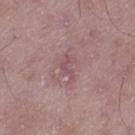Part of a total-body skin-imaging series; this lesion was reviewed on a skin check and was not flagged for biopsy. Longest diameter approximately 2.5 mm. The patient is a male aged 48–52. The lesion-visualizer software estimated a lesion area of about 2.5 mm² and two-axis asymmetry of about 0.6. The analysis additionally found a border-irregularity rating of about 7/10, a color-variation rating of about 0/10, and radial color variation of about 0. And it measured a nevus-likeness score of about 0/100 and a lesion-detection confidence of about 95/100. A 15 mm close-up tile from a total-body photography series done for melanoma screening. Imaged with white-light lighting. On the leg.